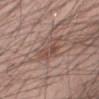No biopsy was performed on this lesion — it was imaged during a full skin examination and was not determined to be concerning. An algorithmic analysis of the crop reported an area of roughly 8 mm², a shape eccentricity near 0.9, and a symmetry-axis asymmetry near 0.4. The analysis additionally found a mean CIELAB color near L≈49 a*≈18 b*≈24, about 8 CIELAB-L* units darker than the surrounding skin, and a normalized lesion–skin contrast near 6. A 15 mm close-up tile from a total-body photography series done for melanoma screening. The subject is a male about 60 years old. Approximately 5 mm at its widest. The tile uses white-light illumination. The lesion is on the mid back.